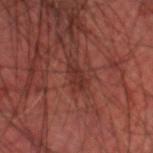Cropped from a whole-body photographic skin survey; the tile spans about 15 mm. Approximately 3.5 mm at its widest. A male subject, aged around 65. The lesion is on the head or neck. This is a cross-polarized tile. Automated tile analysis of the lesion measured a lesion area of about 4 mm² and a shape eccentricity near 0.9. It also reported a border-irregularity rating of about 3.5/10 and radial color variation of about 0.5.